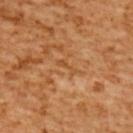The recorded lesion diameter is about 2.5 mm. A roughly 15 mm field-of-view crop from a total-body skin photograph. A female patient about 55 years old. Located on the upper back.